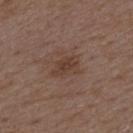The lesion was tiled from a total-body skin photograph and was not biopsied. A 15 mm crop from a total-body photograph taken for skin-cancer surveillance. Captured under white-light illumination. The lesion is on the mid back. The patient is a male approximately 50 years of age.